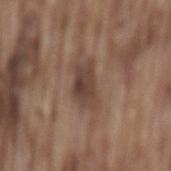Captured during whole-body skin photography for melanoma surveillance; the lesion was not biopsied. The lesion is on the mid back. The tile uses white-light illumination. Automated tile analysis of the lesion measured an area of roughly 8.5 mm², an eccentricity of roughly 0.85, and a symmetry-axis asymmetry near 0.3. It also reported a mean CIELAB color near L≈41 a*≈16 b*≈24 and roughly 10 lightness units darker than nearby skin. The analysis additionally found a border-irregularity rating of about 3.5/10, a color-variation rating of about 3.5/10, and radial color variation of about 1. A male patient aged 73–77. A region of skin cropped from a whole-body photographic capture, roughly 15 mm wide. The recorded lesion diameter is about 4.5 mm.